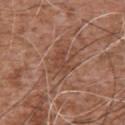The lesion was photographed on a routine skin check and not biopsied; there is no pathology result. Located on the chest. A lesion tile, about 15 mm wide, cut from a 3D total-body photograph. Automated image analysis of the tile measured a footprint of about 6 mm², an outline eccentricity of about 0.75 (0 = round, 1 = elongated), and a shape-asymmetry score of about 0.5 (0 = symmetric). And it measured a mean CIELAB color near L≈45 a*≈22 b*≈28, roughly 7 lightness units darker than nearby skin, and a normalized lesion–skin contrast near 5.5. The analysis additionally found a border-irregularity rating of about 5/10 and a color-variation rating of about 2/10. The software also gave an automated nevus-likeness rating near 0 out of 100 and lesion-presence confidence of about 100/100. This is a white-light tile. A male patient aged 73–77.